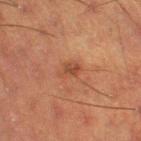workup = no biopsy performed (imaged during a skin exam) | imaging modality = ~15 mm tile from a whole-body skin photo | subject = male, about 60 years old | location = the left thigh | tile lighting = cross-polarized | automated metrics = a border-irregularity index near 3/10, internal color variation of about 2.5 on a 0–10 scale, and peripheral color asymmetry of about 1 | lesion size = ~2.5 mm (longest diameter).